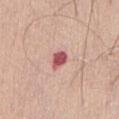Q: Was this lesion biopsied?
A: total-body-photography surveillance lesion; no biopsy
Q: How was the tile lit?
A: white-light illumination
Q: Lesion location?
A: the front of the torso
Q: Lesion size?
A: ~2.5 mm (longest diameter)
Q: What did automated image analysis measure?
A: border irregularity of about 2 on a 0–10 scale and a peripheral color-asymmetry measure near 0.5; a detector confidence of about 100 out of 100 that the crop contains a lesion
Q: What are the patient's age and sex?
A: female, about 55 years old
Q: What kind of image is this?
A: ~15 mm crop, total-body skin-cancer survey